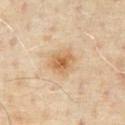{"biopsy_status": "not biopsied; imaged during a skin examination", "site": "chest", "automated_metrics": {"area_mm2_approx": 6.0, "eccentricity": 0.6, "shape_asymmetry": 0.25, "border_irregularity_0_10": 2.5, "color_variation_0_10": 4.0, "peripheral_color_asymmetry": 1.0}, "image": {"source": "total-body photography crop", "field_of_view_mm": 15}, "lesion_size": {"long_diameter_mm_approx": 3.5}, "lighting": "cross-polarized", "patient": {"sex": "male", "age_approx": 65}}